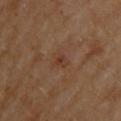histopathology: a nodular basal cell carcinoma (malignant).